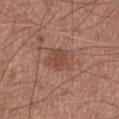The lesion was photographed on a routine skin check and not biopsied; there is no pathology result.
The subject is a male about 75 years old.
The lesion is located on the front of the torso.
Approximately 3.5 mm at its widest.
Imaged with white-light lighting.
Cropped from a total-body skin-imaging series; the visible field is about 15 mm.